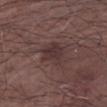follow-up: catalogued during a skin exam; not biopsied | lesion diameter: ≈3.5 mm | body site: the arm | subject: male, aged approximately 70 | acquisition: 15 mm crop, total-body photography | illumination: white-light illumination.